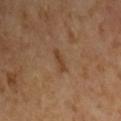Findings:
* subject: male, aged 63–67
* image: ~15 mm tile from a whole-body skin photo